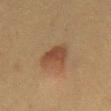Findings:
• patient — female, aged approximately 40
• lighting — cross-polarized
• location — the abdomen
• diameter — ≈3.5 mm
• image source — 15 mm crop, total-body photography